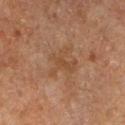body site — the left lower leg
automated lesion analysis — a footprint of about 7.5 mm² and a shape eccentricity near 0.55; roughly 5 lightness units darker than nearby skin and a lesion-to-skin contrast of about 5 (normalized; higher = more distinct); border irregularity of about 8.5 on a 0–10 scale and radial color variation of about 0.5
patient — male, in their mid-60s
tile lighting — cross-polarized
lesion diameter — ≈4 mm
imaging modality — ~15 mm tile from a whole-body skin photo